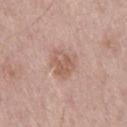On the right thigh. A male patient, aged 73–77. A 15 mm crop from a total-body photograph taken for skin-cancer surveillance. The tile uses white-light illumination.